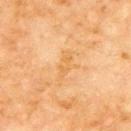image source = ~15 mm tile from a whole-body skin photo
patient = male, in their 70s
tile lighting = cross-polarized illumination
body site = the chest
diameter = ≈4 mm
TBP lesion metrics = a lesion color around L≈56 a*≈18 b*≈40 in CIELAB and a normalized lesion–skin contrast near 5; a nevus-likeness score of about 0/100